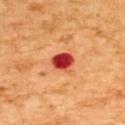  lighting: cross-polarized
  patient:
    sex: male
    age_approx: 60
  site: upper back
  image:
    source: total-body photography crop
    field_of_view_mm: 15